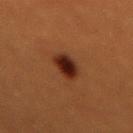tile lighting=cross-polarized illumination
anatomic site=the back
acquisition=~15 mm crop, total-body skin-cancer survey
subject=female, aged around 55
size=about 4 mm
diagnosis=a dysplastic (Clark) nevus